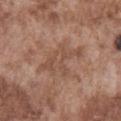<case>
<biopsy_status>not biopsied; imaged during a skin examination</biopsy_status>
<image>
  <source>total-body photography crop</source>
  <field_of_view_mm>15</field_of_view_mm>
</image>
<site>abdomen</site>
<lesion_size>
  <long_diameter_mm_approx>5.5</long_diameter_mm_approx>
</lesion_size>
<automated_metrics>
  <cielab_L>50</cielab_L>
  <cielab_a>19</cielab_a>
  <cielab_b>28</cielab_b>
  <vs_skin_darker_L>7.0</vs_skin_darker_L>
  <vs_skin_contrast_norm>5.0</vs_skin_contrast_norm>
</automated_metrics>
<patient>
  <sex>male</sex>
  <age_approx>75</age_approx>
</patient>
</case>